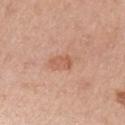workup = catalogued during a skin exam; not biopsied | location = the left upper arm | size = about 2.5 mm | image = ~15 mm crop, total-body skin-cancer survey | patient = female, roughly 45 years of age | lighting = white-light illumination.